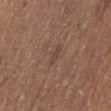Q: Was this lesion biopsied?
A: no biopsy performed (imaged during a skin exam)
Q: Automated lesion metrics?
A: a lesion area of about 2.5 mm², an outline eccentricity of about 0.9 (0 = round, 1 = elongated), and a shape-asymmetry score of about 0.4 (0 = symmetric); a lesion color around L≈43 a*≈18 b*≈23 in CIELAB, about 6 CIELAB-L* units darker than the surrounding skin, and a normalized border contrast of about 5
Q: Where on the body is the lesion?
A: the abdomen
Q: What lighting was used for the tile?
A: white-light
Q: What is the imaging modality?
A: ~15 mm crop, total-body skin-cancer survey
Q: What are the patient's age and sex?
A: male, in their mid-60s
Q: Lesion size?
A: about 2.5 mm